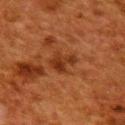  image:
    source: total-body photography crop
    field_of_view_mm: 15
  patient:
    sex: female
    age_approx: 50
  site: back
  lesion_size:
    long_diameter_mm_approx: 3.0